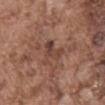lesion diameter: about 3.5 mm; image: total-body-photography crop, ~15 mm field of view; tile lighting: white-light; patient: male, aged 73 to 77; location: the abdomen.